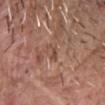Recorded during total-body skin imaging; not selected for excision or biopsy.
Cropped from a total-body skin-imaging series; the visible field is about 15 mm.
A male subject in their mid-60s.
From the head or neck.
Automated image analysis of the tile measured a shape eccentricity near 0.9 and a shape-asymmetry score of about 0.3 (0 = symmetric). It also reported an average lesion color of about L≈49 a*≈20 b*≈27 (CIELAB) and about 6 CIELAB-L* units darker than the surrounding skin. It also reported a border-irregularity index near 3/10, internal color variation of about 4 on a 0–10 scale, and a peripheral color-asymmetry measure near 1.
This is a white-light tile.
About 3 mm across.